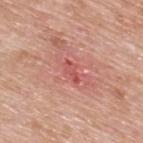<record>
<biopsy_status>not biopsied; imaged during a skin examination</biopsy_status>
<site>upper back</site>
<image>
  <source>total-body photography crop</source>
  <field_of_view_mm>15</field_of_view_mm>
</image>
<patient>
  <sex>male</sex>
  <age_approx>80</age_approx>
</patient>
<lighting>white-light</lighting>
<automated_metrics>
  <area_mm2_approx>2.5</area_mm2_approx>
  <eccentricity>0.9</eccentricity>
  <shape_asymmetry>0.35</shape_asymmetry>
  <nevus_likeness_0_100>0</nevus_likeness_0_100>
</automated_metrics>
</record>